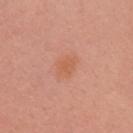Assessment:
Recorded during total-body skin imaging; not selected for excision or biopsy.
Background:
A 15 mm close-up tile from a total-body photography series done for melanoma screening. This is a white-light tile. About 3.5 mm across. The lesion is located on the left upper arm. A female subject, aged around 40.